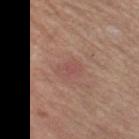Impression:
The lesion was tiled from a total-body skin photograph and was not biopsied.
Background:
About 3 mm across. A female subject about 65 years old. A 15 mm crop from a total-body photograph taken for skin-cancer surveillance. The lesion is on the leg. Imaged with white-light lighting.